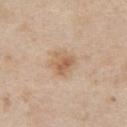Case summary:
– biopsy status · catalogued during a skin exam; not biopsied
– imaging modality · 15 mm crop, total-body photography
– subject · female, aged approximately 45
– site · the chest
– tile lighting · white-light illumination
– lesion diameter · ~3.5 mm (longest diameter)